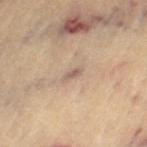Impression:
Imaged during a routine full-body skin examination; the lesion was not biopsied and no histopathology is available.
Background:
Automated image analysis of the tile measured a lesion color around L≈56 a*≈15 b*≈24 in CIELAB, roughly 8 lightness units darker than nearby skin, and a normalized lesion–skin contrast near 6.5. The software also gave border irregularity of about 3 on a 0–10 scale, a within-lesion color-variation index near 0/10, and a peripheral color-asymmetry measure near 0. Captured under cross-polarized illumination. A female subject, aged 63–67. On the left thigh. The recorded lesion diameter is about 2.5 mm. This image is a 15 mm lesion crop taken from a total-body photograph.